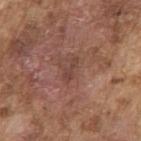Recorded during total-body skin imaging; not selected for excision or biopsy. A male subject, in their mid- to late 70s. The recorded lesion diameter is about 3 mm. The lesion is on the arm. Imaged with white-light lighting. Cropped from a whole-body photographic skin survey; the tile spans about 15 mm.